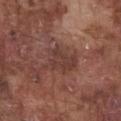biopsy status — catalogued during a skin exam; not biopsied | subject — male, approximately 75 years of age | acquisition — total-body-photography crop, ~15 mm field of view | anatomic site — the chest | TBP lesion metrics — a footprint of about 11 mm² and an outline eccentricity of about 0.65 (0 = round, 1 = elongated); a lesion color around L≈39 a*≈20 b*≈23 in CIELAB and a normalized border contrast of about 6; a within-lesion color-variation index near 3/10 and radial color variation of about 1; an automated nevus-likeness rating near 0 out of 100 and lesion-presence confidence of about 100/100 | lighting — white-light.